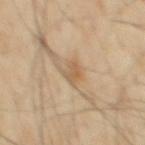biopsy status = no biopsy performed (imaged during a skin exam)
site = the mid back
subject = male, aged 53 to 57
image source = ~15 mm tile from a whole-body skin photo